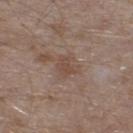Case summary:
• workup · imaged on a skin check; not biopsied
• image source · total-body-photography crop, ~15 mm field of view
• location · the left lower leg
• subject · male, in their mid-40s